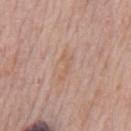workup: total-body-photography surveillance lesion; no biopsy
patient: male, approximately 75 years of age
image source: total-body-photography crop, ~15 mm field of view
anatomic site: the mid back
lesion diameter: ~3 mm (longest diameter)
automated metrics: a footprint of about 3 mm² and an outline eccentricity of about 0.9 (0 = round, 1 = elongated); an automated nevus-likeness rating near 0 out of 100
illumination: white-light